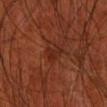Clinical impression:
The lesion was photographed on a routine skin check and not biopsied; there is no pathology result.
Background:
The tile uses cross-polarized illumination. From the front of the torso. Longest diameter approximately 2.5 mm. A male subject about 70 years old. Automated tile analysis of the lesion measured a footprint of about 3 mm² and a shape-asymmetry score of about 0.45 (0 = symmetric). And it measured a lesion color around L≈26 a*≈26 b*≈29 in CIELAB, roughly 6 lightness units darker than nearby skin, and a normalized lesion–skin contrast near 6. The software also gave border irregularity of about 4.5 on a 0–10 scale and a within-lesion color-variation index near 0.5/10. The software also gave a nevus-likeness score of about 0/100 and a lesion-detection confidence of about 95/100. This image is a 15 mm lesion crop taken from a total-body photograph.